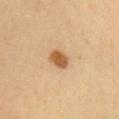{"biopsy_status": "not biopsied; imaged during a skin examination", "lighting": "cross-polarized", "automated_metrics": {"area_mm2_approx": 5.0, "eccentricity": 0.65}, "site": "head or neck", "image": {"source": "total-body photography crop", "field_of_view_mm": 15}, "patient": {"sex": "female", "age_approx": 30}}